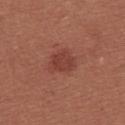No biopsy was performed on this lesion — it was imaged during a full skin examination and was not determined to be concerning.
The total-body-photography lesion software estimated a lesion-detection confidence of about 100/100.
This is a white-light tile.
The patient is a female in their mid-20s.
Cropped from a total-body skin-imaging series; the visible field is about 15 mm.
From the upper back.